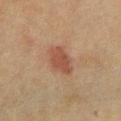biopsy_status: not biopsied; imaged during a skin examination
image:
  source: total-body photography crop
  field_of_view_mm: 15
patient:
  sex: female
  age_approx: 40
site: chest
lesion_size:
  long_diameter_mm_approx: 4.0